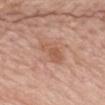An algorithmic analysis of the crop reported a nevus-likeness score of about 0/100 and lesion-presence confidence of about 100/100. Longest diameter approximately 3.5 mm. A female subject, roughly 65 years of age. A close-up tile cropped from a whole-body skin photograph, about 15 mm across. The lesion is located on the mid back.